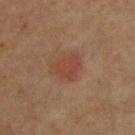- follow-up · total-body-photography surveillance lesion; no biopsy
- tile lighting · cross-polarized illumination
- anatomic site · the upper back
- image source · ~15 mm tile from a whole-body skin photo
- TBP lesion metrics · a footprint of about 7 mm², a shape eccentricity near 0.6, and two-axis asymmetry of about 0.3; a mean CIELAB color near L≈36 a*≈21 b*≈26, roughly 6 lightness units darker than nearby skin, and a lesion-to-skin contrast of about 5.5 (normalized; higher = more distinct); a nevus-likeness score of about 100/100 and a lesion-detection confidence of about 100/100
- subject · male, aged 43–47